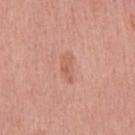Measured at roughly 3.5 mm in maximum diameter. The lesion is on the upper back. A male subject, aged 43 to 47. Cropped from a total-body skin-imaging series; the visible field is about 15 mm.